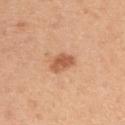This lesion was catalogued during total-body skin photography and was not selected for biopsy. The patient is a female aged 43–47. The lesion is located on the arm. A 15 mm close-up extracted from a 3D total-body photography capture.